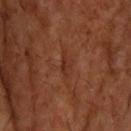Captured during whole-body skin photography for melanoma surveillance; the lesion was not biopsied. On the upper back. This image is a 15 mm lesion crop taken from a total-body photograph. A male subject, roughly 55 years of age.